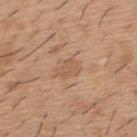From the upper back.
A male subject aged 38–42.
Captured under white-light illumination.
Cropped from a total-body skin-imaging series; the visible field is about 15 mm.
Longest diameter approximately 3 mm.